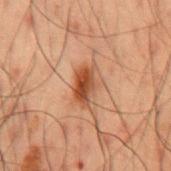Acquisition and patient details:
This is a cross-polarized tile. A male patient in their 50s. Approximately 4 mm at its widest. The lesion is on the mid back. Automated tile analysis of the lesion measured a lesion area of about 6.5 mm² and an eccentricity of roughly 0.85. The software also gave a mean CIELAB color near L≈37 a*≈21 b*≈29 and about 11 CIELAB-L* units darker than the surrounding skin. A 15 mm close-up extracted from a 3D total-body photography capture.